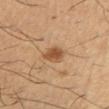| key | value |
|---|---|
| image source | total-body-photography crop, ~15 mm field of view |
| lesion diameter | ~3 mm (longest diameter) |
| patient | male, approximately 40 years of age |
| illumination | cross-polarized |
| location | the right upper arm |